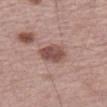The lesion was tiled from a total-body skin photograph and was not biopsied.